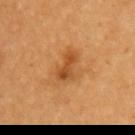<tbp_lesion>
<lesion_size>
  <long_diameter_mm_approx>3.5</long_diameter_mm_approx>
</lesion_size>
<patient>
  <sex>female</sex>
  <age_approx>30</age_approx>
</patient>
<image>
  <source>total-body photography crop</source>
  <field_of_view_mm>15</field_of_view_mm>
</image>
<lighting>cross-polarized</lighting>
<site>back</site>
</tbp_lesion>